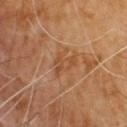Q: What did automated image analysis measure?
A: a footprint of about 4 mm², an eccentricity of roughly 0.8, and a symmetry-axis asymmetry near 0.6; a border-irregularity rating of about 7/10, a color-variation rating of about 2.5/10, and radial color variation of about 0.5
Q: What is the lesion's diameter?
A: ≈3.5 mm
Q: What kind of image is this?
A: ~15 mm crop, total-body skin-cancer survey
Q: Where on the body is the lesion?
A: the upper back
Q: What are the patient's age and sex?
A: male, aged around 60